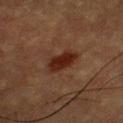{
  "image": {
    "source": "total-body photography crop",
    "field_of_view_mm": 15
  },
  "site": "back",
  "lesion_size": {
    "long_diameter_mm_approx": 4.5
  },
  "patient": {
    "sex": "male",
    "age_approx": 55
  },
  "lighting": "cross-polarized"
}